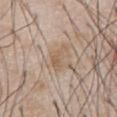Context: Longest diameter approximately 4 mm. The tile uses white-light illumination. The lesion is located on the abdomen. The patient is a male roughly 60 years of age. A 15 mm crop from a total-body photograph taken for skin-cancer surveillance.